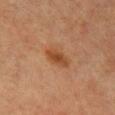biopsy status: imaged on a skin check; not biopsied
image source: ~15 mm crop, total-body skin-cancer survey
site: the chest
patient: female, aged 43 to 47